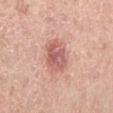  patient:
    sex: female
    age_approx: 65
  image:
    source: total-body photography crop
    field_of_view_mm: 15
  lighting: white-light
  site: leg
  automated_metrics:
    cielab_L: 59
    cielab_a: 27
    cielab_b: 26
    vs_skin_darker_L: 12.0
    vs_skin_contrast_norm: 7.5
    color_variation_0_10: 4.5
    peripheral_color_asymmetry: 1.5